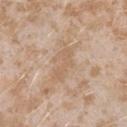| key | value |
|---|---|
| site | the left forearm |
| acquisition | ~15 mm tile from a whole-body skin photo |
| tile lighting | white-light illumination |
| subject | female, aged around 25 |
| diameter | ~4 mm (longest diameter) |